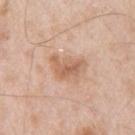Findings:
- notes — imaged on a skin check; not biopsied
- location — the left upper arm
- TBP lesion metrics — an area of roughly 7 mm² and a shape-asymmetry score of about 0.5 (0 = symmetric); a mean CIELAB color near L≈61 a*≈21 b*≈32 and about 10 CIELAB-L* units darker than the surrounding skin; border irregularity of about 5.5 on a 0–10 scale and peripheral color asymmetry of about 1
- lesion diameter — about 4 mm
- image source — ~15 mm tile from a whole-body skin photo
- tile lighting — white-light illumination
- subject — male, about 55 years old